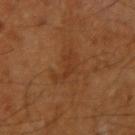{"biopsy_status": "not biopsied; imaged during a skin examination", "patient": {"sex": "male", "age_approx": 60}, "site": "right upper arm", "image": {"source": "total-body photography crop", "field_of_view_mm": 15}, "lighting": "cross-polarized"}